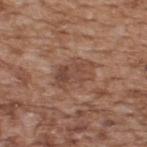  biopsy_status: not biopsied; imaged during a skin examination
  image:
    source: total-body photography crop
    field_of_view_mm: 15
  patient:
    sex: male
    age_approx: 65
  lighting: white-light
  automated_metrics:
    cielab_L: 46
    cielab_a: 20
    cielab_b: 27
    vs_skin_contrast_norm: 6.0
    border_irregularity_0_10: 3.5
    color_variation_0_10: 4.5
    peripheral_color_asymmetry: 1.5
  site: upper back